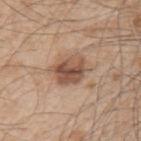Q: Was this lesion biopsied?
A: no biopsy performed (imaged during a skin exam)
Q: How was the tile lit?
A: white-light illumination
Q: What kind of image is this?
A: total-body-photography crop, ~15 mm field of view
Q: Lesion location?
A: the left upper arm
Q: How large is the lesion?
A: about 4 mm
Q: What did automated image analysis measure?
A: about 13 CIELAB-L* units darker than the surrounding skin and a normalized border contrast of about 9
Q: Who is the patient?
A: male, aged 48 to 52